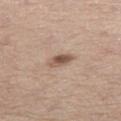| feature | finding |
|---|---|
| follow-up | no biopsy performed (imaged during a skin exam) |
| image | ~15 mm crop, total-body skin-cancer survey |
| patient | female, roughly 65 years of age |
| image-analysis metrics | border irregularity of about 2 on a 0–10 scale, a color-variation rating of about 5/10, and a peripheral color-asymmetry measure near 1.5; a nevus-likeness score of about 80/100 and a lesion-detection confidence of about 100/100 |
| site | the leg |
| diameter | ~3 mm (longest diameter) |